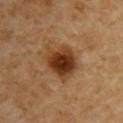Recorded during total-body skin imaging; not selected for excision or biopsy. The subject is a male approximately 60 years of age. From the left upper arm. A region of skin cropped from a whole-body photographic capture, roughly 15 mm wide.